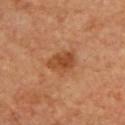Notes:
- workup — total-body-photography surveillance lesion; no biopsy
- subject — female, in their mid-50s
- diameter — about 4 mm
- automated lesion analysis — an eccentricity of roughly 0.75; a mean CIELAB color near L≈49 a*≈26 b*≈39, a lesion–skin lightness drop of about 10, and a normalized border contrast of about 7.5; a nevus-likeness score of about 60/100 and a lesion-detection confidence of about 100/100
- image — total-body-photography crop, ~15 mm field of view
- illumination — cross-polarized
- anatomic site — the upper back